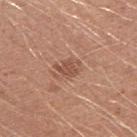notes=total-body-photography surveillance lesion; no biopsy.